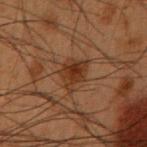biopsy status: imaged on a skin check; not biopsied
subject: male, aged around 55
illumination: cross-polarized
body site: the arm
automated lesion analysis: a footprint of about 7.5 mm² and an eccentricity of roughly 0.5; a border-irregularity rating of about 4/10, a color-variation rating of about 4/10, and radial color variation of about 1.5
image source: ~15 mm crop, total-body skin-cancer survey
diameter: ≈3.5 mm